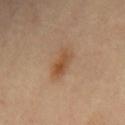{"lighting": "cross-polarized", "site": "back", "automated_metrics": {"area_mm2_approx": 7.0, "shape_asymmetry": 0.3, "vs_skin_darker_L": 9.0}, "image": {"source": "total-body photography crop", "field_of_view_mm": 15}}